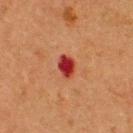Clinical impression:
Imaged during a routine full-body skin examination; the lesion was not biopsied and no histopathology is available.